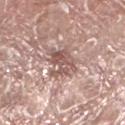Assessment: Imaged during a routine full-body skin examination; the lesion was not biopsied and no histopathology is available. Image and clinical context: Cropped from a total-body skin-imaging series; the visible field is about 15 mm. A male subject, roughly 75 years of age. Captured under white-light illumination. The total-body-photography lesion software estimated an average lesion color of about L≈55 a*≈20 b*≈23 (CIELAB). The analysis additionally found border irregularity of about 5.5 on a 0–10 scale, a color-variation rating of about 5.5/10, and peripheral color asymmetry of about 2. The lesion is located on the left lower leg. Measured at roughly 4 mm in maximum diameter.